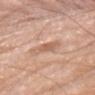Clinical summary:
An algorithmic analysis of the crop reported an outline eccentricity of about 0.65 (0 = round, 1 = elongated). It also reported an average lesion color of about L≈61 a*≈21 b*≈32 (CIELAB), a lesion–skin lightness drop of about 9, and a normalized border contrast of about 6. It also reported a border-irregularity rating of about 2.5/10, internal color variation of about 2.5 on a 0–10 scale, and radial color variation of about 1. The analysis additionally found a classifier nevus-likeness of about 0/100 and lesion-presence confidence of about 100/100. Measured at roughly 3 mm in maximum diameter. A male patient, approximately 80 years of age. From the right upper arm. Cropped from a whole-body photographic skin survey; the tile spans about 15 mm. The tile uses white-light illumination.